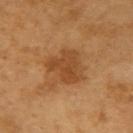The lesion was tiled from a total-body skin photograph and was not biopsied.
A 15 mm close-up tile from a total-body photography series done for melanoma screening.
The tile uses cross-polarized illumination.
From the right upper arm.
The subject is a male aged approximately 65.
Automated tile analysis of the lesion measured border irregularity of about 4 on a 0–10 scale, internal color variation of about 2.5 on a 0–10 scale, and a peripheral color-asymmetry measure near 1. And it measured a classifier nevus-likeness of about 15/100 and a lesion-detection confidence of about 100/100.
About 4 mm across.